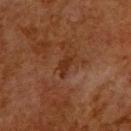Assessment: Recorded during total-body skin imaging; not selected for excision or biopsy. Image and clinical context: This is a cross-polarized tile. A 15 mm close-up extracted from a 3D total-body photography capture. From the upper back. The patient is a male aged around 65.